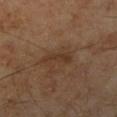| field | value |
|---|---|
| notes | total-body-photography surveillance lesion; no biopsy |
| anatomic site | the right lower leg |
| image | ~15 mm tile from a whole-body skin photo |
| patient | male, in their 70s |
| lighting | cross-polarized illumination |